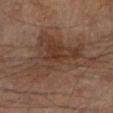– TBP lesion metrics — a lesion area of about 31 mm²; a mean CIELAB color near L≈37 a*≈16 b*≈25, a lesion–skin lightness drop of about 8, and a normalized lesion–skin contrast near 7; a border-irregularity index near 8/10 and a peripheral color-asymmetry measure near 1.5
– location — the leg
– diameter — about 10 mm
– image source — ~15 mm tile from a whole-body skin photo
– patient — male, about 70 years old
– lighting — cross-polarized